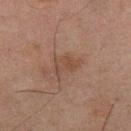follow-up: catalogued during a skin exam; not biopsied
location: the right thigh
lighting: cross-polarized illumination
subject: male, aged approximately 65
size: about 3 mm
image: ~15 mm tile from a whole-body skin photo